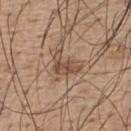Notes:
• biopsy status: catalogued during a skin exam; not biopsied
• image-analysis metrics: an average lesion color of about L≈50 a*≈17 b*≈29 (CIELAB), roughly 10 lightness units darker than nearby skin, and a normalized lesion–skin contrast near 7; a border-irregularity index near 6/10, internal color variation of about 3.5 on a 0–10 scale, and radial color variation of about 1.5
• anatomic site: the upper back
• subject: male, roughly 55 years of age
• tile lighting: white-light
• lesion diameter: ~4 mm (longest diameter)
• image: 15 mm crop, total-body photography